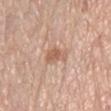workup: catalogued during a skin exam; not biopsied | acquisition: ~15 mm tile from a whole-body skin photo | anatomic site: the front of the torso | patient: female, in their mid- to late 60s.